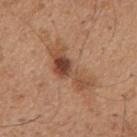biopsy status=no biopsy performed (imaged during a skin exam).